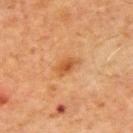Impression:
The lesion was tiled from a total-body skin photograph and was not biopsied.
Context:
The subject is a male about 70 years old. A 15 mm close-up tile from a total-body photography series done for melanoma screening. On the arm. About 3.5 mm across. An algorithmic analysis of the crop reported a footprint of about 5 mm² and a symmetry-axis asymmetry near 0.25. It also reported a mean CIELAB color near L≈46 a*≈22 b*≈36, about 8 CIELAB-L* units darker than the surrounding skin, and a lesion-to-skin contrast of about 7 (normalized; higher = more distinct). The software also gave a nevus-likeness score of about 45/100. The tile uses cross-polarized illumination.